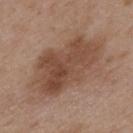Notes:
- workup · total-body-photography surveillance lesion; no biopsy
- TBP lesion metrics · an area of roughly 37 mm², a shape eccentricity near 0.85, and a symmetry-axis asymmetry near 0.2; a lesion-detection confidence of about 100/100
- anatomic site · the upper back
- imaging modality · ~15 mm crop, total-body skin-cancer survey
- tile lighting · white-light
- subject · male, about 50 years old